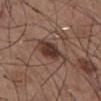<lesion>
<biopsy_status>not biopsied; imaged during a skin examination</biopsy_status>
<lighting>white-light</lighting>
<site>abdomen</site>
<lesion_size>
  <long_diameter_mm_approx>4.0</long_diameter_mm_approx>
</lesion_size>
<automated_metrics>
  <cielab_L>37</cielab_L>
  <cielab_a>18</cielab_a>
  <cielab_b>23</cielab_b>
  <vs_skin_darker_L>11.0</vs_skin_darker_L>
  <vs_skin_contrast_norm>9.5</vs_skin_contrast_norm>
</automated_metrics>
<image>
  <source>total-body photography crop</source>
  <field_of_view_mm>15</field_of_view_mm>
</image>
<patient>
  <sex>male</sex>
  <age_approx>55</age_approx>
</patient>
</lesion>